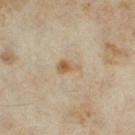| key | value |
|---|---|
| notes | no biopsy performed (imaged during a skin exam) |
| lesion diameter | ~2.5 mm (longest diameter) |
| lighting | cross-polarized |
| acquisition | ~15 mm crop, total-body skin-cancer survey |
| anatomic site | the right thigh |
| patient | female, aged 33–37 |